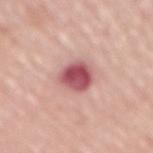Part of a total-body skin-imaging series; this lesion was reviewed on a skin check and was not flagged for biopsy. Captured under white-light illumination. The lesion-visualizer software estimated an area of roughly 9 mm² and two-axis asymmetry of about 0.2. The lesion is on the abdomen. Approximately 3.5 mm at its widest. A roughly 15 mm field-of-view crop from a total-body skin photograph. A female subject, in their mid- to late 60s.